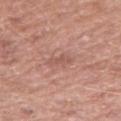Captured during whole-body skin photography for melanoma surveillance; the lesion was not biopsied.
Automated tile analysis of the lesion measured an area of roughly 3.5 mm², an outline eccentricity of about 0.85 (0 = round, 1 = elongated), and two-axis asymmetry of about 0.35. The software also gave a border-irregularity rating of about 4/10, internal color variation of about 1 on a 0–10 scale, and a peripheral color-asymmetry measure near 0.5. And it measured an automated nevus-likeness rating near 0 out of 100 and a detector confidence of about 100 out of 100 that the crop contains a lesion.
Approximately 3 mm at its widest.
The lesion is on the right forearm.
A 15 mm close-up extracted from a 3D total-body photography capture.
A male subject, in their 60s.